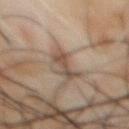Recorded during total-body skin imaging; not selected for excision or biopsy.
A roughly 15 mm field-of-view crop from a total-body skin photograph.
The lesion is located on the chest.
A male subject aged 48–52.